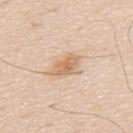workup = total-body-photography surveillance lesion; no biopsy
body site = the upper back
image source = total-body-photography crop, ~15 mm field of view
lighting = white-light
patient = male, roughly 60 years of age